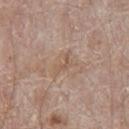Q: Is there a histopathology result?
A: catalogued during a skin exam; not biopsied
Q: What is the imaging modality?
A: ~15 mm tile from a whole-body skin photo
Q: How was the tile lit?
A: white-light illumination
Q: Patient demographics?
A: male, aged approximately 65
Q: Where on the body is the lesion?
A: the chest
Q: How large is the lesion?
A: about 3 mm
Q: What did automated image analysis measure?
A: a border-irregularity rating of about 6.5/10, a color-variation rating of about 0/10, and radial color variation of about 0